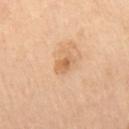Clinical impression: The lesion was photographed on a routine skin check and not biopsied; there is no pathology result. Image and clinical context: The patient is a female aged around 65. A close-up tile cropped from a whole-body skin photograph, about 15 mm across. About 3 mm across. Located on the right thigh. Captured under cross-polarized illumination.